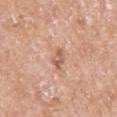This lesion was catalogued during total-body skin photography and was not selected for biopsy. The lesion is located on the left upper arm. Longest diameter approximately 2.5 mm. Cropped from a total-body skin-imaging series; the visible field is about 15 mm. A female subject aged approximately 60. Imaged with white-light lighting.